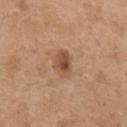  biopsy_status: not biopsied; imaged during a skin examination
  lesion_size:
    long_diameter_mm_approx: 3.0
  patient:
    sex: female
    age_approx: 30
  image:
    source: total-body photography crop
    field_of_view_mm: 15
  site: right upper arm
  lighting: white-light